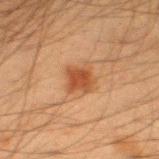Q: Was a biopsy performed?
A: total-body-photography surveillance lesion; no biopsy
Q: What lighting was used for the tile?
A: cross-polarized
Q: What did automated image analysis measure?
A: a footprint of about 6 mm², an eccentricity of roughly 0.5, and a shape-asymmetry score of about 0.2 (0 = symmetric); an average lesion color of about L≈39 a*≈21 b*≈31 (CIELAB), a lesion–skin lightness drop of about 9, and a lesion-to-skin contrast of about 8 (normalized; higher = more distinct); a classifier nevus-likeness of about 95/100 and a lesion-detection confidence of about 100/100
Q: What is the anatomic site?
A: the left thigh
Q: Who is the patient?
A: male, in their mid-30s
Q: What kind of image is this?
A: ~15 mm tile from a whole-body skin photo
Q: What is the lesion's diameter?
A: about 3 mm